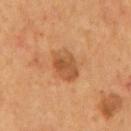notes: catalogued during a skin exam; not biopsied
automated lesion analysis: a lesion area of about 8.5 mm², a shape eccentricity near 0.8, and two-axis asymmetry of about 0.15; a lesion color around L≈48 a*≈22 b*≈36 in CIELAB, about 9 CIELAB-L* units darker than the surrounding skin, and a lesion-to-skin contrast of about 7 (normalized; higher = more distinct); a border-irregularity index near 1.5/10, a within-lesion color-variation index near 4/10, and radial color variation of about 1.5
patient: male, aged 53 to 57
location: the mid back
acquisition: total-body-photography crop, ~15 mm field of view
lesion size: ~4 mm (longest diameter)